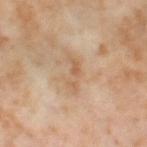Clinical impression: Imaged during a routine full-body skin examination; the lesion was not biopsied and no histopathology is available. Clinical summary: A female subject aged around 55. The lesion-visualizer software estimated a nevus-likeness score of about 0/100. A lesion tile, about 15 mm wide, cut from a 3D total-body photograph. From the right thigh. Imaged with cross-polarized lighting.